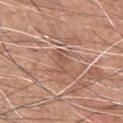Q: Was a biopsy performed?
A: imaged on a skin check; not biopsied
Q: Lesion size?
A: about 3 mm
Q: Who is the patient?
A: male, about 60 years old
Q: What kind of image is this?
A: 15 mm crop, total-body photography
Q: Automated lesion metrics?
A: a footprint of about 3 mm² and a shape-asymmetry score of about 0.35 (0 = symmetric); a border-irregularity index near 5/10, a color-variation rating of about 0.5/10, and peripheral color asymmetry of about 0; a classifier nevus-likeness of about 0/100 and a lesion-detection confidence of about 55/100
Q: What is the anatomic site?
A: the chest
Q: How was the tile lit?
A: white-light illumination